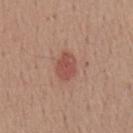{
  "biopsy_status": "not biopsied; imaged during a skin examination",
  "site": "chest",
  "lighting": "white-light",
  "patient": {
    "sex": "male",
    "age_approx": 55
  },
  "image": {
    "source": "total-body photography crop",
    "field_of_view_mm": 15
  },
  "automated_metrics": {
    "vs_skin_darker_L": 10.0,
    "vs_skin_contrast_norm": 7.0,
    "lesion_detection_confidence_0_100": 100
  },
  "lesion_size": {
    "long_diameter_mm_approx": 3.5
  }
}